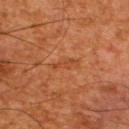Impression:
Captured during whole-body skin photography for melanoma surveillance; the lesion was not biopsied.
Image and clinical context:
This image is a 15 mm lesion crop taken from a total-body photograph. Located on the back. Captured under cross-polarized illumination. Automated image analysis of the tile measured border irregularity of about 4 on a 0–10 scale, internal color variation of about 0 on a 0–10 scale, and a peripheral color-asymmetry measure near 0. A male patient aged 63–67. About 2.5 mm across.